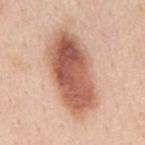Q: Was a biopsy performed?
A: total-body-photography surveillance lesion; no biopsy
Q: What did automated image analysis measure?
A: a lesion area of about 36 mm² and a shape-asymmetry score of about 0.15 (0 = symmetric); a border-irregularity rating of about 2/10 and a color-variation rating of about 8/10; a detector confidence of about 100 out of 100 that the crop contains a lesion
Q: Who is the patient?
A: male, aged 48–52
Q: How was this image acquired?
A: total-body-photography crop, ~15 mm field of view
Q: How large is the lesion?
A: ~10 mm (longest diameter)
Q: What is the anatomic site?
A: the mid back